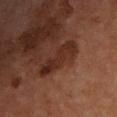This lesion was catalogued during total-body skin photography and was not selected for biopsy.
The patient is a male in their mid- to late 60s.
The lesion-visualizer software estimated an area of roughly 9.5 mm², an outline eccentricity of about 0.95 (0 = round, 1 = elongated), and a shape-asymmetry score of about 0.25 (0 = symmetric). It also reported a mean CIELAB color near L≈27 a*≈21 b*≈25, a lesion–skin lightness drop of about 7, and a normalized border contrast of about 8.
The lesion is located on the upper back.
Approximately 5.5 mm at its widest.
A 15 mm close-up tile from a total-body photography series done for melanoma screening.